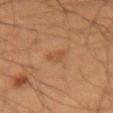The lesion was photographed on a routine skin check and not biopsied; there is no pathology result.
From the right forearm.
A 15 mm close-up extracted from a 3D total-body photography capture.
A male subject aged approximately 35.